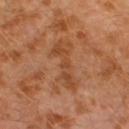<record>
<patient>
  <sex>male</sex>
  <age_approx>30</age_approx>
</patient>
<site>left lower leg</site>
<image>
  <source>total-body photography crop</source>
  <field_of_view_mm>15</field_of_view_mm>
</image>
<lighting>cross-polarized</lighting>
<automated_metrics>
  <cielab_L>45</cielab_L>
  <cielab_a>23</cielab_a>
  <cielab_b>35</cielab_b>
  <vs_skin_darker_L>7.0</vs_skin_darker_L>
  <vs_skin_contrast_norm>6.0</vs_skin_contrast_norm>
  <color_variation_0_10>2.5</color_variation_0_10>
  <peripheral_color_asymmetry>0.5</peripheral_color_asymmetry>
  <nevus_likeness_0_100>0</nevus_likeness_0_100>
  <lesion_detection_confidence_0_100>90</lesion_detection_confidence_0_100>
</automated_metrics>
<lesion_size>
  <long_diameter_mm_approx>6.0</long_diameter_mm_approx>
</lesion_size>
</record>